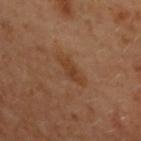Part of a total-body skin-imaging series; this lesion was reviewed on a skin check and was not flagged for biopsy. This is a cross-polarized tile. A female patient, about 60 years old. A lesion tile, about 15 mm wide, cut from a 3D total-body photograph. An algorithmic analysis of the crop reported a footprint of about 5.5 mm², an outline eccentricity of about 0.9 (0 = round, 1 = elongated), and two-axis asymmetry of about 0.3. The software also gave a normalized lesion–skin contrast near 6. The analysis additionally found lesion-presence confidence of about 100/100. Measured at roughly 4 mm in maximum diameter. The lesion is located on the upper back.